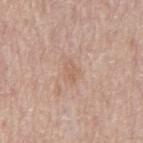patient: male, aged 78 to 82
image: 15 mm crop, total-body photography
site: the back